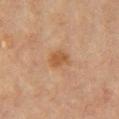Imaged during a routine full-body skin examination; the lesion was not biopsied and no histopathology is available. A female patient aged around 65. The tile uses cross-polarized illumination. About 3 mm across. A roughly 15 mm field-of-view crop from a total-body skin photograph. An algorithmic analysis of the crop reported an eccentricity of roughly 0.65 and a symmetry-axis asymmetry near 0.15. It also reported an average lesion color of about L≈46 a*≈19 b*≈33 (CIELAB), about 7 CIELAB-L* units darker than the surrounding skin, and a lesion-to-skin contrast of about 7.5 (normalized; higher = more distinct). The software also gave a border-irregularity rating of about 1.5/10, a within-lesion color-variation index near 2/10, and radial color variation of about 1. And it measured a classifier nevus-likeness of about 55/100. On the front of the torso.